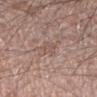Case summary:
– follow-up: total-body-photography surveillance lesion; no biopsy
– anatomic site: the left forearm
– illumination: white-light
– TBP lesion metrics: an average lesion color of about L≈53 a*≈17 b*≈23 (CIELAB), about 6 CIELAB-L* units darker than the surrounding skin, and a normalized border contrast of about 4.5; a border-irregularity index near 3/10, a color-variation rating of about 1.5/10, and peripheral color asymmetry of about 0.5
– acquisition: 15 mm crop, total-body photography
– patient: male, about 55 years old
– diameter: about 2.5 mm